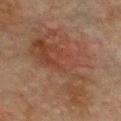Approximately 11.5 mm at its widest.
A 15 mm crop from a total-body photograph taken for skin-cancer surveillance.
A male patient roughly 75 years of age.
The lesion is on the chest.